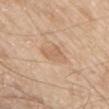Q: Was a biopsy performed?
A: imaged on a skin check; not biopsied
Q: Lesion location?
A: the chest
Q: What is the lesion's diameter?
A: ~3.5 mm (longest diameter)
Q: What are the patient's age and sex?
A: male, roughly 80 years of age
Q: Illumination type?
A: white-light illumination
Q: Automated lesion metrics?
A: an area of roughly 6 mm², an eccentricity of roughly 0.7, and two-axis asymmetry of about 0.25; about 8 CIELAB-L* units darker than the surrounding skin and a normalized border contrast of about 5.5; a border-irregularity rating of about 2.5/10, a within-lesion color-variation index near 1.5/10, and radial color variation of about 0.5
Q: What is the imaging modality?
A: ~15 mm tile from a whole-body skin photo